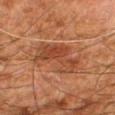Part of a total-body skin-imaging series; this lesion was reviewed on a skin check and was not flagged for biopsy.
The tile uses cross-polarized illumination.
On the right thigh.
A 15 mm close-up extracted from a 3D total-body photography capture.
A male patient, aged around 80.
An algorithmic analysis of the crop reported an area of roughly 14 mm², a shape eccentricity near 0.8, and a symmetry-axis asymmetry near 0.5.